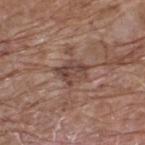Measured at roughly 3.5 mm in maximum diameter.
A 15 mm close-up tile from a total-body photography series done for melanoma screening.
The lesion is on the upper back.
A male patient approximately 70 years of age.